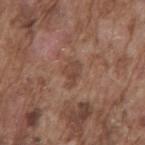Impression: This lesion was catalogued during total-body skin photography and was not selected for biopsy. Acquisition and patient details: From the mid back. A close-up tile cropped from a whole-body skin photograph, about 15 mm across. A male patient aged 73 to 77.